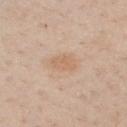Case summary:
• follow-up — total-body-photography surveillance lesion; no biopsy
• tile lighting — white-light illumination
• diameter — ≈3 mm
• subject — male, aged 38 to 42
• acquisition — 15 mm crop, total-body photography
• anatomic site — the chest